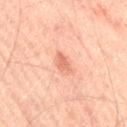biopsy_status: not biopsied; imaged during a skin examination
lesion_size:
  long_diameter_mm_approx: 2.5
site: right thigh
patient:
  sex: male
  age_approx: 65
lighting: cross-polarized
image:
  source: total-body photography crop
  field_of_view_mm: 15